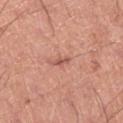Assessment:
No biopsy was performed on this lesion — it was imaged during a full skin examination and was not determined to be concerning.
Background:
A 15 mm close-up extracted from a 3D total-body photography capture. From the right lower leg. The subject is a male in their mid- to late 60s. Imaged with white-light lighting.